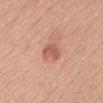Notes:
• biopsy status: imaged on a skin check; not biopsied
• acquisition: ~15 mm crop, total-body skin-cancer survey
• site: the front of the torso
• diameter: ~2.5 mm (longest diameter)
• image-analysis metrics: a mean CIELAB color near L≈57 a*≈27 b*≈31 and a normalized lesion–skin contrast near 7; border irregularity of about 3 on a 0–10 scale, a color-variation rating of about 2.5/10, and radial color variation of about 1
• subject: female, in their 40s